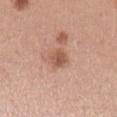No biopsy was performed on this lesion — it was imaged during a full skin examination and was not determined to be concerning. The total-body-photography lesion software estimated a shape eccentricity near 0.65 and a shape-asymmetry score of about 0.15 (0 = symmetric). The software also gave an automated nevus-likeness rating near 30 out of 100 and a detector confidence of about 100 out of 100 that the crop contains a lesion. Captured under white-light illumination. The subject is a female aged 28 to 32. Cropped from a total-body skin-imaging series; the visible field is about 15 mm. From the left upper arm. About 2.5 mm across.